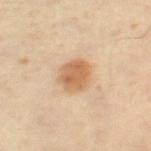  lighting: cross-polarized
  image:
    source: total-body photography crop
    field_of_view_mm: 15
  site: right thigh
  automated_metrics:
    area_mm2_approx: 8.5
    shape_asymmetry: 0.15
    cielab_L: 58
    cielab_a: 19
    cielab_b: 35
    vs_skin_darker_L: 11.0
    vs_skin_contrast_norm: 8.0
    color_variation_0_10: 3.5
    peripheral_color_asymmetry: 1.0
  patient:
    sex: male
    age_approx: 60
  lesion_size:
    long_diameter_mm_approx: 4.0